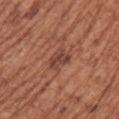workup — total-body-photography surveillance lesion; no biopsy
subject — female, in their mid- to late 70s
image source — total-body-photography crop, ~15 mm field of view
diameter — about 3 mm
illumination — white-light
location — the left upper arm